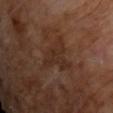Assessment:
The lesion was tiled from a total-body skin photograph and was not biopsied.
Background:
The lesion-visualizer software estimated a lesion area of about 10 mm², a shape eccentricity near 0.75, and a shape-asymmetry score of about 0.6 (0 = symmetric). And it measured a lesion color around L≈27 a*≈17 b*≈24 in CIELAB, a lesion–skin lightness drop of about 4, and a lesion-to-skin contrast of about 5 (normalized; higher = more distinct). It also reported border irregularity of about 7 on a 0–10 scale, internal color variation of about 2 on a 0–10 scale, and a peripheral color-asymmetry measure near 1. Cropped from a total-body skin-imaging series; the visible field is about 15 mm. A male subject, aged around 60. Located on the upper back. Measured at roughly 5.5 mm in maximum diameter.